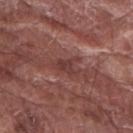lighting = white-light; location = the right forearm; imaging modality = total-body-photography crop, ~15 mm field of view; subject = male, approximately 75 years of age.